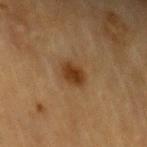This lesion was catalogued during total-body skin photography and was not selected for biopsy. The recorded lesion diameter is about 3 mm. On the arm. The subject is a male in their mid-80s or older. A close-up tile cropped from a whole-body skin photograph, about 15 mm across. Captured under cross-polarized illumination.